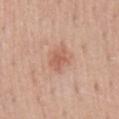An algorithmic analysis of the crop reported a lesion area of about 5.5 mm², a shape eccentricity near 0.7, and a symmetry-axis asymmetry near 0.3. And it measured a mean CIELAB color near L≈59 a*≈24 b*≈30 and a normalized lesion–skin contrast near 6. The software also gave border irregularity of about 3 on a 0–10 scale and a peripheral color-asymmetry measure near 0.5. It also reported a nevus-likeness score of about 45/100 and a lesion-detection confidence of about 100/100.
On the back.
Longest diameter approximately 3 mm.
A roughly 15 mm field-of-view crop from a total-body skin photograph.
Imaged with white-light lighting.
A male patient aged approximately 55.